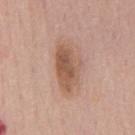Assessment:
This lesion was catalogued during total-body skin photography and was not selected for biopsy.
Acquisition and patient details:
Imaged with white-light lighting. The lesion is on the chest. A 15 mm crop from a total-body photograph taken for skin-cancer surveillance. A male subject aged 53–57. Measured at roughly 6 mm in maximum diameter.